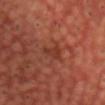follow-up=no biopsy performed (imaged during a skin exam); subject=male, roughly 55 years of age; location=the head or neck; size=about 2.5 mm; image=total-body-photography crop, ~15 mm field of view.